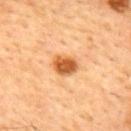No biopsy was performed on this lesion — it was imaged during a full skin examination and was not determined to be concerning. A 15 mm crop from a total-body photograph taken for skin-cancer surveillance. The lesion is located on the upper back. A male subject aged 43 to 47.